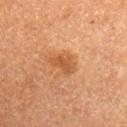This lesion was catalogued during total-body skin photography and was not selected for biopsy. Cropped from a total-body skin-imaging series; the visible field is about 15 mm. Located on the left upper arm. The total-body-photography lesion software estimated a lesion color around L≈48 a*≈23 b*≈37 in CIELAB, about 8 CIELAB-L* units darker than the surrounding skin, and a normalized border contrast of about 6.5. It also reported border irregularity of about 3 on a 0–10 scale and a within-lesion color-variation index near 2/10. The lesion's longest dimension is about 3.5 mm. Imaged with cross-polarized lighting. The subject is a female roughly 55 years of age.